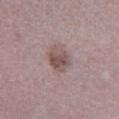Recorded during total-body skin imaging; not selected for excision or biopsy. The tile uses white-light illumination. This image is a 15 mm lesion crop taken from a total-body photograph. The subject is a male aged approximately 50. Automated tile analysis of the lesion measured a border-irregularity rating of about 1.5/10 and a color-variation rating of about 3.5/10. It also reported a detector confidence of about 100 out of 100 that the crop contains a lesion. Longest diameter approximately 3.5 mm. From the left lower leg.